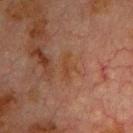A male subject, about 80 years old.
Captured under cross-polarized illumination.
The lesion's longest dimension is about 3 mm.
On the chest.
Cropped from a total-body skin-imaging series; the visible field is about 15 mm.